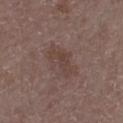From the left thigh. The subject is a female aged around 85 or older. This is a white-light tile. This image is a 15 mm lesion crop taken from a total-body photograph. Automated tile analysis of the lesion measured a lesion area of about 8.5 mm², an outline eccentricity of about 0.9 (0 = round, 1 = elongated), and a shape-asymmetry score of about 0.35 (0 = symmetric). And it measured roughly 6 lightness units darker than nearby skin and a normalized border contrast of about 5.5. It also reported a within-lesion color-variation index near 2.5/10 and peripheral color asymmetry of about 1. The analysis additionally found a detector confidence of about 100 out of 100 that the crop contains a lesion. Measured at roughly 4.5 mm in maximum diameter.